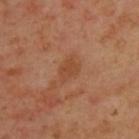Q: Is there a histopathology result?
A: no biopsy performed (imaged during a skin exam)
Q: What is the lesion's diameter?
A: about 3 mm
Q: What is the imaging modality?
A: 15 mm crop, total-body photography
Q: What did automated image analysis measure?
A: a border-irregularity rating of about 2.5/10, a color-variation rating of about 1.5/10, and radial color variation of about 0.5
Q: What lighting was used for the tile?
A: cross-polarized
Q: Who is the patient?
A: male, aged approximately 45
Q: Lesion location?
A: the back